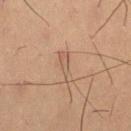* workup: total-body-photography surveillance lesion; no biopsy
* diameter: ≈3.5 mm
* body site: the leg
* automated lesion analysis: a lesion area of about 4 mm², a shape eccentricity near 0.95, and a symmetry-axis asymmetry near 0.35; border irregularity of about 4.5 on a 0–10 scale, a within-lesion color-variation index near 1.5/10, and a peripheral color-asymmetry measure near 0; a nevus-likeness score of about 0/100 and a lesion-detection confidence of about 100/100
* subject: male, about 55 years old
* tile lighting: cross-polarized
* acquisition: 15 mm crop, total-body photography